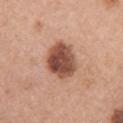Notes:
- lesion diameter · ~4.5 mm (longest diameter)
- automated metrics · a lesion area of about 14 mm², an eccentricity of roughly 0.6, and a shape-asymmetry score of about 0.15 (0 = symmetric)
- patient · female, about 60 years old
- image · ~15 mm crop, total-body skin-cancer survey
- tile lighting · white-light illumination
- location · the right upper arm